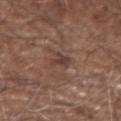Imaged during a routine full-body skin examination; the lesion was not biopsied and no histopathology is available.
Cropped from a whole-body photographic skin survey; the tile spans about 15 mm.
The lesion is located on the left forearm.
The tile uses white-light illumination.
The lesion-visualizer software estimated a footprint of about 3.5 mm², a shape eccentricity near 0.75, and a symmetry-axis asymmetry near 0.35. The analysis additionally found a lesion–skin lightness drop of about 8. And it measured border irregularity of about 3.5 on a 0–10 scale, a within-lesion color-variation index near 2/10, and a peripheral color-asymmetry measure near 0.5. The software also gave a detector confidence of about 95 out of 100 that the crop contains a lesion.
A male patient, in their mid- to late 70s.